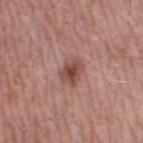Clinical summary: Automated image analysis of the tile measured an area of roughly 6.5 mm² and an eccentricity of roughly 0.65. The analysis additionally found a mean CIELAB color near L≈48 a*≈23 b*≈25 and a lesion–skin lightness drop of about 11. And it measured a border-irregularity rating of about 2/10, internal color variation of about 5 on a 0–10 scale, and a peripheral color-asymmetry measure near 1.5. The analysis additionally found an automated nevus-likeness rating near 85 out of 100 and a detector confidence of about 100 out of 100 that the crop contains a lesion. A male subject, aged 53 to 57. This image is a 15 mm lesion crop taken from a total-body photograph. Imaged with white-light lighting. About 3.5 mm across. On the right thigh.